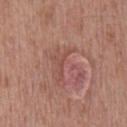Impression: No biopsy was performed on this lesion — it was imaged during a full skin examination and was not determined to be concerning. Context: The tile uses white-light illumination. A region of skin cropped from a whole-body photographic capture, roughly 15 mm wide. From the back. A male patient, aged 58–62. Approximately 3.5 mm at its widest.